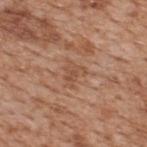Notes:
• subject · male, aged 63 to 67
• site · the upper back
• automated metrics · border irregularity of about 4.5 on a 0–10 scale, a color-variation rating of about 2/10, and a peripheral color-asymmetry measure near 0.5
• diameter · about 2.5 mm
• acquisition · ~15 mm tile from a whole-body skin photo